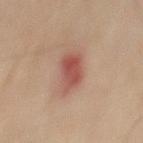<lesion>
  <biopsy_status>not biopsied; imaged during a skin examination</biopsy_status>
  <lighting>cross-polarized</lighting>
  <patient>
    <sex>male</sex>
    <age_approx>60</age_approx>
  </patient>
  <image>
    <source>total-body photography crop</source>
    <field_of_view_mm>15</field_of_view_mm>
  </image>
  <site>mid back</site>
  <lesion_size>
    <long_diameter_mm_approx>4.5</long_diameter_mm_approx>
  </lesion_size>
  <automated_metrics>
    <eccentricity>0.85</eccentricity>
    <border_irregularity_0_10>3.0</border_irregularity_0_10>
    <color_variation_0_10>3.5</color_variation_0_10>
  </automated_metrics>
</lesion>